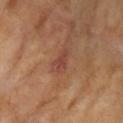biopsy status: total-body-photography surveillance lesion; no biopsy
image: 15 mm crop, total-body photography
location: the right upper arm
illumination: cross-polarized illumination
patient: female, aged 73 to 77
automated lesion analysis: a footprint of about 5 mm², a shape eccentricity near 0.75, and two-axis asymmetry of about 0.3; an average lesion color of about L≈44 a*≈24 b*≈29 (CIELAB), about 7 CIELAB-L* units darker than the surrounding skin, and a normalized lesion–skin contrast near 6